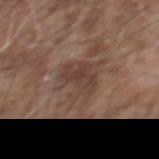| feature | finding |
|---|---|
| patient | male, aged around 55 |
| imaging modality | ~15 mm tile from a whole-body skin photo |
| lighting | white-light illumination |
| anatomic site | the back |